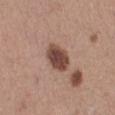Captured during whole-body skin photography for melanoma surveillance; the lesion was not biopsied.
The subject is a female aged 33–37.
The lesion is located on the right lower leg.
The tile uses white-light illumination.
A region of skin cropped from a whole-body photographic capture, roughly 15 mm wide.